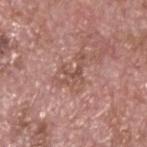Assessment:
No biopsy was performed on this lesion — it was imaged during a full skin examination and was not determined to be concerning.
Clinical summary:
The lesion is on the head or neck. A region of skin cropped from a whole-body photographic capture, roughly 15 mm wide. An algorithmic analysis of the crop reported a classifier nevus-likeness of about 0/100 and lesion-presence confidence of about 95/100. The subject is a male roughly 75 years of age. This is a white-light tile.